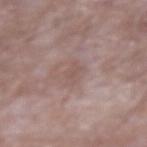  biopsy_status: not biopsied; imaged during a skin examination
  patient:
    sex: male
    age_approx: 65
  site: right upper arm
  lesion_size:
    long_diameter_mm_approx: 2.5
  image:
    source: total-body photography crop
    field_of_view_mm: 15